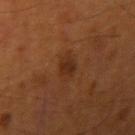{"biopsy_status": "not biopsied; imaged during a skin examination", "site": "right upper arm", "patient": {"sex": "male", "age_approx": 55}, "lesion_size": {"long_diameter_mm_approx": 2.5}, "image": {"source": "total-body photography crop", "field_of_view_mm": 15}}